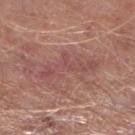subject: male, aged around 65 | body site: the leg | image source: ~15 mm tile from a whole-body skin photo.